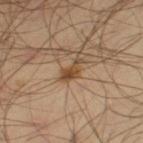This lesion was catalogued during total-body skin photography and was not selected for biopsy.